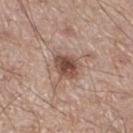Q: Was a biopsy performed?
A: total-body-photography surveillance lesion; no biopsy
Q: Lesion location?
A: the right thigh
Q: What is the lesion's diameter?
A: ≈3.5 mm
Q: How was the tile lit?
A: white-light illumination
Q: What kind of image is this?
A: 15 mm crop, total-body photography
Q: Who is the patient?
A: male, aged 58 to 62